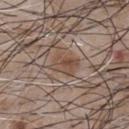biopsy status = no biopsy performed (imaged during a skin exam)
image-analysis metrics = a lesion–skin lightness drop of about 8 and a normalized border contrast of about 6.5; border irregularity of about 3 on a 0–10 scale and internal color variation of about 4.5 on a 0–10 scale
subject = male, about 50 years old
image = total-body-photography crop, ~15 mm field of view
anatomic site = the chest
tile lighting = white-light illumination